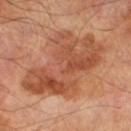Image and clinical context:
This is a cross-polarized tile. The lesion is on the left lower leg. A region of skin cropped from a whole-body photographic capture, roughly 15 mm wide. The patient is a male in their 70s. The lesion-visualizer software estimated an average lesion color of about L≈50 a*≈25 b*≈33 (CIELAB), roughly 10 lightness units darker than nearby skin, and a lesion-to-skin contrast of about 7 (normalized; higher = more distinct). Measured at roughly 9.5 mm in maximum diameter.